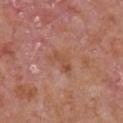Part of a total-body skin-imaging series; this lesion was reviewed on a skin check and was not flagged for biopsy. A region of skin cropped from a whole-body photographic capture, roughly 15 mm wide. This is a white-light tile. The subject is a male aged approximately 65. An algorithmic analysis of the crop reported a footprint of about 5 mm² and a symmetry-axis asymmetry near 0.5. The software also gave a lesion color around L≈51 a*≈24 b*≈31 in CIELAB, about 6 CIELAB-L* units darker than the surrounding skin, and a lesion-to-skin contrast of about 6 (normalized; higher = more distinct). And it measured border irregularity of about 5.5 on a 0–10 scale and radial color variation of about 0.5. Located on the chest. The lesion's longest dimension is about 3.5 mm.